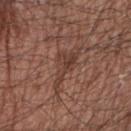<lesion>
  <biopsy_status>not biopsied; imaged during a skin examination</biopsy_status>
  <patient>
    <sex>male</sex>
    <age_approx>65</age_approx>
  </patient>
  <site>right upper arm</site>
  <image>
    <source>total-body photography crop</source>
    <field_of_view_mm>15</field_of_view_mm>
  </image>
  <automated_metrics>
    <area_mm2_approx>7.5</area_mm2_approx>
    <eccentricity>0.9</eccentricity>
    <shape_asymmetry>0.55</shape_asymmetry>
    <vs_skin_darker_L>8.0</vs_skin_darker_L>
    <vs_skin_contrast_norm>6.5</vs_skin_contrast_norm>
    <color_variation_0_10>3.0</color_variation_0_10>
    <peripheral_color_asymmetry>1.0</peripheral_color_asymmetry>
    <nevus_likeness_0_100>0</nevus_likeness_0_100>
    <lesion_detection_confidence_0_100>85</lesion_detection_confidence_0_100>
  </automated_metrics>
</lesion>